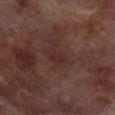Part of a total-body skin-imaging series; this lesion was reviewed on a skin check and was not flagged for biopsy.